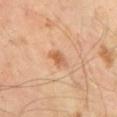This image is a 15 mm lesion crop taken from a total-body photograph. A male patient approximately 65 years of age. Captured under cross-polarized illumination. The lesion-visualizer software estimated a footprint of about 3.5 mm² and a symmetry-axis asymmetry near 0.35. It also reported a border-irregularity rating of about 3.5/10, a within-lesion color-variation index near 2/10, and radial color variation of about 0.5. The lesion's longest dimension is about 3 mm.